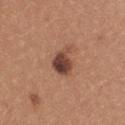Q: Was a biopsy performed?
A: imaged on a skin check; not biopsied
Q: What is the imaging modality?
A: 15 mm crop, total-body photography
Q: How was the tile lit?
A: white-light
Q: How large is the lesion?
A: about 3.5 mm
Q: Where on the body is the lesion?
A: the upper back
Q: What are the patient's age and sex?
A: female, aged around 20